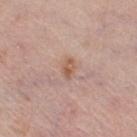Longest diameter approximately 2.5 mm. Captured under white-light illumination. The lesion is on the right thigh. The subject is a female aged 43–47. This image is a 15 mm lesion crop taken from a total-body photograph.